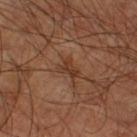* workup · no biopsy performed (imaged during a skin exam)
* acquisition · ~15 mm tile from a whole-body skin photo
* location · the left lower leg
* patient · male, aged approximately 60